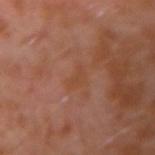Q: Was this lesion biopsied?
A: no biopsy performed (imaged during a skin exam)
Q: What is the anatomic site?
A: the left arm
Q: Automated lesion metrics?
A: a color-variation rating of about 0/10 and peripheral color asymmetry of about 0; a classifier nevus-likeness of about 0/100
Q: Patient demographics?
A: male, aged 28 to 32
Q: What is the lesion's diameter?
A: ~2.5 mm (longest diameter)
Q: What is the imaging modality?
A: ~15 mm tile from a whole-body skin photo